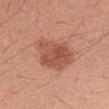Q: Was this lesion biopsied?
A: imaged on a skin check; not biopsied
Q: Automated lesion metrics?
A: a mean CIELAB color near L≈53 a*≈26 b*≈31, roughly 11 lightness units darker than nearby skin, and a normalized lesion–skin contrast near 7.5
Q: Who is the patient?
A: female, aged around 40
Q: Lesion location?
A: the left forearm
Q: What is the imaging modality?
A: ~15 mm tile from a whole-body skin photo
Q: Lesion size?
A: ~4.5 mm (longest diameter)